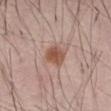Q: Was this lesion biopsied?
A: imaged on a skin check; not biopsied
Q: Where on the body is the lesion?
A: the abdomen
Q: Patient demographics?
A: male, in their 60s
Q: What is the imaging modality?
A: total-body-photography crop, ~15 mm field of view